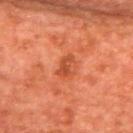The lesion was photographed on a routine skin check and not biopsied; there is no pathology result. The tile uses cross-polarized illumination. A region of skin cropped from a whole-body photographic capture, roughly 15 mm wide. A male patient, aged 63 to 67. Measured at roughly 2.5 mm in maximum diameter. The lesion is located on the back.